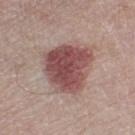Part of a total-body skin-imaging series; this lesion was reviewed on a skin check and was not flagged for biopsy. Located on the right thigh. Cropped from a whole-body photographic skin survey; the tile spans about 15 mm. The subject is a male roughly 80 years of age.